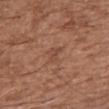workup = imaged on a skin check; not biopsied
image source = ~15 mm crop, total-body skin-cancer survey
TBP lesion metrics = an automated nevus-likeness rating near 0 out of 100 and a detector confidence of about 100 out of 100 that the crop contains a lesion
illumination = white-light illumination
location = the right upper arm
patient = male, approximately 75 years of age